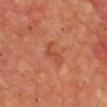Clinical impression: Recorded during total-body skin imaging; not selected for excision or biopsy. Clinical summary: The patient is a male aged 68 to 72. This image is a 15 mm lesion crop taken from a total-body photograph. Automated image analysis of the tile measured an average lesion color of about L≈40 a*≈25 b*≈29 (CIELAB), roughly 5 lightness units darker than nearby skin, and a normalized border contrast of about 5. And it measured border irregularity of about 6 on a 0–10 scale, internal color variation of about 1 on a 0–10 scale, and peripheral color asymmetry of about 0.5. The recorded lesion diameter is about 3.5 mm. On the chest.